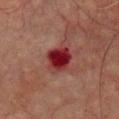follow-up=catalogued during a skin exam; not biopsied
illumination=cross-polarized
automated metrics=a footprint of about 9.5 mm² and a shape eccentricity near 0.55; a mean CIELAB color near L≈27 a*≈31 b*≈21, roughly 13 lightness units darker than nearby skin, and a normalized lesion–skin contrast near 12.5; a border-irregularity rating of about 1.5/10 and internal color variation of about 4 on a 0–10 scale; a detector confidence of about 100 out of 100 that the crop contains a lesion
location=the chest
patient=male, in their mid- to late 70s
image=~15 mm crop, total-body skin-cancer survey
lesion size=~3.5 mm (longest diameter)